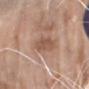No biopsy was performed on this lesion — it was imaged during a full skin examination and was not determined to be concerning. A female subject approximately 70 years of age. The lesion is on the right forearm. This is a white-light tile. A 15 mm close-up tile from a total-body photography series done for melanoma screening. Measured at roughly 4 mm in maximum diameter.